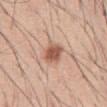Captured during whole-body skin photography for melanoma surveillance; the lesion was not biopsied. The lesion-visualizer software estimated a footprint of about 6 mm² and an outline eccentricity of about 0.7 (0 = round, 1 = elongated). The software also gave a color-variation rating of about 3.5/10. Approximately 3.5 mm at its widest. Captured under white-light illumination. The patient is a male approximately 45 years of age. On the abdomen. A region of skin cropped from a whole-body photographic capture, roughly 15 mm wide.